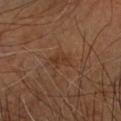Clinical impression: No biopsy was performed on this lesion — it was imaged during a full skin examination and was not determined to be concerning. Acquisition and patient details: The lesion is on the right upper arm. The patient is a male aged around 60. The recorded lesion diameter is about 2.5 mm. Cropped from a total-body skin-imaging series; the visible field is about 15 mm. Automated tile analysis of the lesion measured a lesion color around L≈32 a*≈19 b*≈29 in CIELAB and a normalized lesion–skin contrast near 6.